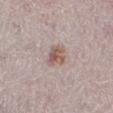Case summary:
- notes: catalogued during a skin exam; not biopsied
- lesion size: ~2.5 mm (longest diameter)
- subject: female, aged around 50
- location: the left lower leg
- automated lesion analysis: a mean CIELAB color near L≈56 a*≈16 b*≈23, roughly 11 lightness units darker than nearby skin, and a lesion-to-skin contrast of about 8 (normalized; higher = more distinct); border irregularity of about 2 on a 0–10 scale, internal color variation of about 4 on a 0–10 scale, and peripheral color asymmetry of about 1.5
- image source: total-body-photography crop, ~15 mm field of view
- tile lighting: white-light